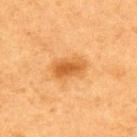Imaged during a routine full-body skin examination; the lesion was not biopsied and no histopathology is available.
The subject is a female aged around 40.
Cropped from a whole-body photographic skin survey; the tile spans about 15 mm.
The lesion is located on the upper back.